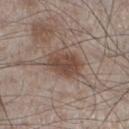– follow-up — no biopsy performed (imaged during a skin exam)
– imaging modality — 15 mm crop, total-body photography
– site — the left lower leg
– subject — male, aged 58–62